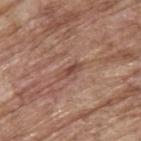This lesion was catalogued during total-body skin photography and was not selected for biopsy. From the upper back. A male patient roughly 70 years of age. A 15 mm close-up tile from a total-body photography series done for melanoma screening.